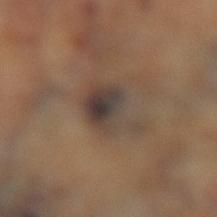Recorded during total-body skin imaging; not selected for excision or biopsy. This is a cross-polarized tile. The lesion-visualizer software estimated a mean CIELAB color near L≈41 a*≈12 b*≈21, roughly 9 lightness units darker than nearby skin, and a normalized lesion–skin contrast near 9.5. The software also gave a border-irregularity rating of about 4.5/10, a color-variation rating of about 9/10, and radial color variation of about 2.5. A region of skin cropped from a whole-body photographic capture, roughly 15 mm wide. Located on the leg.